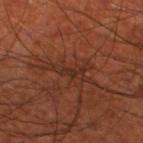notes: imaged on a skin check; not biopsied
automated lesion analysis: a lesion–skin lightness drop of about 6 and a lesion-to-skin contrast of about 6 (normalized; higher = more distinct); border irregularity of about 10 on a 0–10 scale, internal color variation of about 2.5 on a 0–10 scale, and peripheral color asymmetry of about 0.5; an automated nevus-likeness rating near 0 out of 100 and lesion-presence confidence of about 75/100
lighting: cross-polarized
diameter: ~5.5 mm (longest diameter)
image: total-body-photography crop, ~15 mm field of view
patient: male, approximately 70 years of age
site: the left lower leg